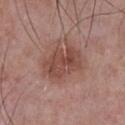Q: What is the lesion's diameter?
A: ~5 mm (longest diameter)
Q: Lesion location?
A: the chest
Q: What did automated image analysis measure?
A: a lesion area of about 14 mm² and two-axis asymmetry of about 0.2; an average lesion color of about L≈46 a*≈23 b*≈24 (CIELAB), about 9 CIELAB-L* units darker than the surrounding skin, and a lesion-to-skin contrast of about 7 (normalized; higher = more distinct)
Q: How was this image acquired?
A: total-body-photography crop, ~15 mm field of view
Q: Illumination type?
A: white-light illumination
Q: What are the patient's age and sex?
A: male, in their mid-60s